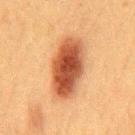The lesion was photographed on a routine skin check and not biopsied; there is no pathology result. About 7 mm across. Captured under cross-polarized illumination. A male subject, in their mid-30s. A close-up tile cropped from a whole-body skin photograph, about 15 mm across. The lesion-visualizer software estimated an area of roughly 22 mm² and an eccentricity of roughly 0.85. And it measured an automated nevus-likeness rating near 100 out of 100 and lesion-presence confidence of about 100/100. From the mid back.